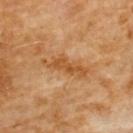follow-up: imaged on a skin check; not biopsied | patient: male, in their 60s | acquisition: ~15 mm tile from a whole-body skin photo | site: the chest | lighting: cross-polarized illumination | lesion size: ~5.5 mm (longest diameter).